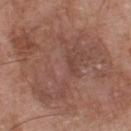Part of a total-body skin-imaging series; this lesion was reviewed on a skin check and was not flagged for biopsy. Located on the chest. A roughly 15 mm field-of-view crop from a total-body skin photograph. A male subject, aged approximately 55. Imaged with white-light lighting. An algorithmic analysis of the crop reported a footprint of about 95 mm², an outline eccentricity of about 0.75 (0 = round, 1 = elongated), and two-axis asymmetry of about 0.4. The software also gave an average lesion color of about L≈49 a*≈20 b*≈26 (CIELAB), about 8 CIELAB-L* units darker than the surrounding skin, and a lesion-to-skin contrast of about 6 (normalized; higher = more distinct). The analysis additionally found a border-irregularity index near 7/10 and internal color variation of about 6 on a 0–10 scale. Longest diameter approximately 15 mm.